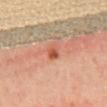Clinical impression:
Part of a total-body skin-imaging series; this lesion was reviewed on a skin check and was not flagged for biopsy.
Context:
A female subject, aged 48 to 52. Captured under cross-polarized illumination. The lesion is on the back. A region of skin cropped from a whole-body photographic capture, roughly 15 mm wide. Approximately 2 mm at its widest.